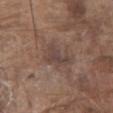Context: Located on the abdomen. A 15 mm crop from a total-body photograph taken for skin-cancer surveillance. A male patient in their 80s. The tile uses white-light illumination.